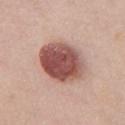{"biopsy_status": "not biopsied; imaged during a skin examination", "automated_metrics": {"area_mm2_approx": 22.0, "shape_asymmetry": 0.1, "cielab_L": 52, "cielab_a": 24, "cielab_b": 24, "vs_skin_darker_L": 19.0, "vs_skin_contrast_norm": 12.0, "border_irregularity_0_10": 1.0, "nevus_likeness_0_100": 95}, "image": {"source": "total-body photography crop", "field_of_view_mm": 15}, "lesion_size": {"long_diameter_mm_approx": 6.5}, "patient": {"sex": "female", "age_approx": 60}, "lighting": "white-light", "site": "chest"}